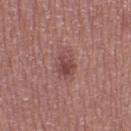Recorded during total-body skin imaging; not selected for excision or biopsy.
The total-body-photography lesion software estimated an average lesion color of about L≈45 a*≈23 b*≈22 (CIELAB) and a lesion-to-skin contrast of about 7 (normalized; higher = more distinct). It also reported an automated nevus-likeness rating near 60 out of 100 and a detector confidence of about 100 out of 100 that the crop contains a lesion.
Captured under white-light illumination.
The lesion is located on the right thigh.
A 15 mm crop from a total-body photograph taken for skin-cancer surveillance.
A female subject, aged around 40.